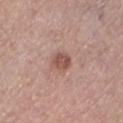The lesion was tiled from a total-body skin photograph and was not biopsied. Imaged with white-light lighting. From the left lower leg. Longest diameter approximately 2.5 mm. An algorithmic analysis of the crop reported a lesion area of about 4.5 mm², a shape eccentricity near 0.65, and two-axis asymmetry of about 0.2. It also reported a nevus-likeness score of about 60/100 and a detector confidence of about 100 out of 100 that the crop contains a lesion. A lesion tile, about 15 mm wide, cut from a 3D total-body photograph. A male subject aged 53–57.